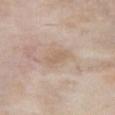No biopsy was performed on this lesion — it was imaged during a full skin examination and was not determined to be concerning. The subject is a female in their mid-60s. Longest diameter approximately 3.5 mm. A close-up tile cropped from a whole-body skin photograph, about 15 mm across. The tile uses white-light illumination. The lesion is on the chest.